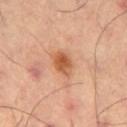Clinical impression: The lesion was tiled from a total-body skin photograph and was not biopsied. Clinical summary: The lesion is located on the left thigh. Automated image analysis of the tile measured an average lesion color of about L≈59 a*≈26 b*≈39 (CIELAB), a lesion–skin lightness drop of about 12, and a normalized lesion–skin contrast near 8.5. It also reported a within-lesion color-variation index near 4.5/10 and a peripheral color-asymmetry measure near 1.5. About 3 mm across. Cropped from a whole-body photographic skin survey; the tile spans about 15 mm.